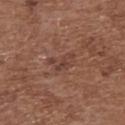No biopsy was performed on this lesion — it was imaged during a full skin examination and was not determined to be concerning. A 15 mm close-up tile from a total-body photography series done for melanoma screening. A female subject, aged 73–77. Automated tile analysis of the lesion measured a footprint of about 5.5 mm² and an outline eccentricity of about 0.8 (0 = round, 1 = elongated). It also reported a border-irregularity rating of about 5/10, a color-variation rating of about 3/10, and radial color variation of about 1. On the upper back. The tile uses white-light illumination. The lesion's longest dimension is about 3.5 mm.